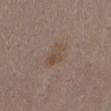Captured during whole-body skin photography for melanoma surveillance; the lesion was not biopsied. A 15 mm close-up tile from a total-body photography series done for melanoma screening. On the front of the torso. A female subject roughly 30 years of age.